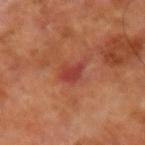No biopsy was performed on this lesion — it was imaged during a full skin examination and was not determined to be concerning. A close-up tile cropped from a whole-body skin photograph, about 15 mm across. The lesion's longest dimension is about 2.5 mm. On the right forearm. The tile uses cross-polarized illumination. A male subject about 55 years old.